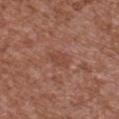Context:
A male patient in their mid- to late 40s. Approximately 2.5 mm at its widest. A 15 mm crop from a total-body photograph taken for skin-cancer surveillance. From the chest.